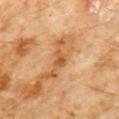Assessment: The lesion was photographed on a routine skin check and not biopsied; there is no pathology result. Acquisition and patient details: From the chest. A lesion tile, about 15 mm wide, cut from a 3D total-body photograph. The tile uses cross-polarized illumination. A male subject about 60 years old.